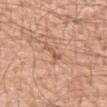biopsy status — imaged on a skin check; not biopsied | anatomic site — the left forearm | subject — male, aged approximately 50 | image source — total-body-photography crop, ~15 mm field of view.